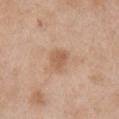The lesion was tiled from a total-body skin photograph and was not biopsied. This image is a 15 mm lesion crop taken from a total-body photograph. The lesion's longest dimension is about 2.5 mm. The patient is a female in their 40s. On the right upper arm.